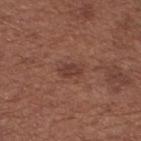| feature | finding |
|---|---|
| notes | catalogued during a skin exam; not biopsied |
| patient | female, about 55 years old |
| imaging modality | total-body-photography crop, ~15 mm field of view |
| illumination | white-light |
| location | the right thigh |